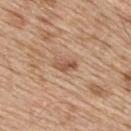Captured during whole-body skin photography for melanoma surveillance; the lesion was not biopsied. Automated tile analysis of the lesion measured a lesion area of about 3.5 mm², an outline eccentricity of about 0.9 (0 = round, 1 = elongated), and a symmetry-axis asymmetry near 0.35. The software also gave a lesion color around L≈54 a*≈21 b*≈31 in CIELAB, a lesion–skin lightness drop of about 11, and a normalized border contrast of about 7.5. The lesion is located on the mid back. Imaged with white-light lighting. A male patient, aged 68 to 72. About 3 mm across. A 15 mm close-up extracted from a 3D total-body photography capture.